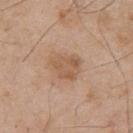A close-up tile cropped from a whole-body skin photograph, about 15 mm across.
The patient is a male aged approximately 55.
From the upper back.